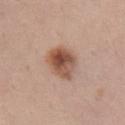Approximately 4.5 mm at its widest. From the chest. Automated tile analysis of the lesion measured a lesion color around L≈52 a*≈21 b*≈29 in CIELAB, roughly 14 lightness units darker than nearby skin, and a normalized lesion–skin contrast near 9.5. It also reported a border-irregularity rating of about 2/10, a within-lesion color-variation index near 8.5/10, and radial color variation of about 3. Cropped from a total-body skin-imaging series; the visible field is about 15 mm. A female patient, approximately 35 years of age.